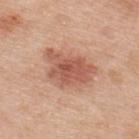| key | value |
|---|---|
| lesion size | ~6 mm (longest diameter) |
| acquisition | 15 mm crop, total-body photography |
| automated metrics | an area of roughly 18 mm², an outline eccentricity of about 0.7 (0 = round, 1 = elongated), and a shape-asymmetry score of about 0.3 (0 = symmetric); a lesion color around L≈57 a*≈24 b*≈30 in CIELAB and a normalized lesion–skin contrast near 7.5 |
| tile lighting | white-light illumination |
| subject | male, approximately 50 years of age |
| site | the back |